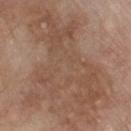workup: total-body-photography surveillance lesion; no biopsy.